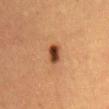Imaged during a routine full-body skin examination; the lesion was not biopsied and no histopathology is available. A female patient, aged approximately 55. The lesion is on the mid back. A close-up tile cropped from a whole-body skin photograph, about 15 mm across. The recorded lesion diameter is about 3 mm. The tile uses cross-polarized illumination. Automated image analysis of the tile measured an area of roughly 4.5 mm², an outline eccentricity of about 0.75 (0 = round, 1 = elongated), and two-axis asymmetry of about 0.2.